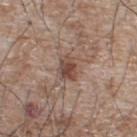| feature | finding |
|---|---|
| follow-up | catalogued during a skin exam; not biopsied |
| acquisition | ~15 mm tile from a whole-body skin photo |
| site | the back |
| subject | male, aged approximately 65 |